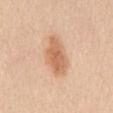Captured during whole-body skin photography for melanoma surveillance; the lesion was not biopsied.
This is a white-light tile.
About 5 mm across.
A region of skin cropped from a whole-body photographic capture, roughly 15 mm wide.
A female subject, roughly 40 years of age.
On the chest.